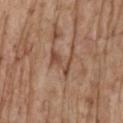Impression:
No biopsy was performed on this lesion — it was imaged during a full skin examination and was not determined to be concerning.
Clinical summary:
A 15 mm close-up tile from a total-body photography series done for melanoma screening. Measured at roughly 3.5 mm in maximum diameter. On the mid back. The total-body-photography lesion software estimated a shape eccentricity near 0.75 and a shape-asymmetry score of about 0.5 (0 = symmetric). And it measured a lesion color around L≈48 a*≈20 b*≈30 in CIELAB and a normalized lesion–skin contrast near 6.5. It also reported an automated nevus-likeness rating near 0 out of 100 and lesion-presence confidence of about 85/100. The subject is a male about 70 years old. Imaged with white-light lighting.